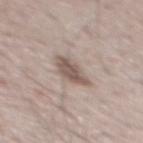Q: Was a biopsy performed?
A: imaged on a skin check; not biopsied
Q: Where on the body is the lesion?
A: the mid back
Q: Automated lesion metrics?
A: a lesion color around L≈54 a*≈14 b*≈22 in CIELAB, about 12 CIELAB-L* units darker than the surrounding skin, and a normalized border contrast of about 8.5; lesion-presence confidence of about 95/100
Q: Illumination type?
A: white-light
Q: What is the lesion's diameter?
A: ~3.5 mm (longest diameter)
Q: How was this image acquired?
A: ~15 mm crop, total-body skin-cancer survey
Q: What are the patient's age and sex?
A: male, aged 68 to 72